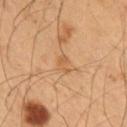| field | value |
|---|---|
| workup | catalogued during a skin exam; not biopsied |